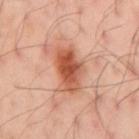Clinical impression: Captured during whole-body skin photography for melanoma surveillance; the lesion was not biopsied. Acquisition and patient details: The recorded lesion diameter is about 5 mm. This is a cross-polarized tile. The subject is a male aged 53 to 57. On the mid back. Cropped from a whole-body photographic skin survey; the tile spans about 15 mm. The total-body-photography lesion software estimated a lesion area of about 12 mm², a shape eccentricity near 0.75, and a symmetry-axis asymmetry near 0.25. The software also gave border irregularity of about 2.5 on a 0–10 scale, a color-variation rating of about 6/10, and a peripheral color-asymmetry measure near 1.5. The software also gave a classifier nevus-likeness of about 95/100.